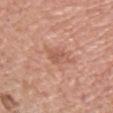Clinical impression:
Captured during whole-body skin photography for melanoma surveillance; the lesion was not biopsied.
Image and clinical context:
The patient is a male approximately 75 years of age. Longest diameter approximately 2.5 mm. A region of skin cropped from a whole-body photographic capture, roughly 15 mm wide. The lesion is located on the chest.